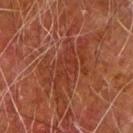Imaged during a routine full-body skin examination; the lesion was not biopsied and no histopathology is available.